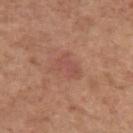Background:
The lesion's longest dimension is about 3.5 mm. A female patient aged 63 to 67. The tile uses white-light illumination. From the arm. A roughly 15 mm field-of-view crop from a total-body skin photograph.